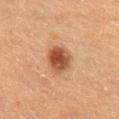<record>
  <biopsy_status>not biopsied; imaged during a skin examination</biopsy_status>
  <site>lower back</site>
  <patient>
    <sex>female</sex>
    <age_approx>55</age_approx>
  </patient>
  <image>
    <source>total-body photography crop</source>
    <field_of_view_mm>15</field_of_view_mm>
  </image>
  <lighting>cross-polarized</lighting>
</record>